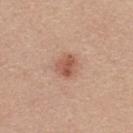Recorded during total-body skin imaging; not selected for excision or biopsy.
On the upper back.
A roughly 15 mm field-of-view crop from a total-body skin photograph.
The subject is a female approximately 35 years of age.
Captured under white-light illumination.